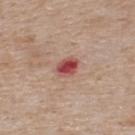The lesion's longest dimension is about 3 mm. The patient is a male in their mid-70s. From the upper back. The lesion-visualizer software estimated an area of roughly 4.5 mm², a shape eccentricity near 0.65, and a shape-asymmetry score of about 0.15 (0 = symmetric). The analysis additionally found a lesion color around L≈49 a*≈31 b*≈25 in CIELAB and a lesion-to-skin contrast of about 10 (normalized; higher = more distinct). The software also gave a border-irregularity index near 1.5/10, internal color variation of about 5 on a 0–10 scale, and a peripheral color-asymmetry measure near 2. The analysis additionally found a nevus-likeness score of about 0/100. Cropped from a whole-body photographic skin survey; the tile spans about 15 mm. Imaged with white-light lighting.